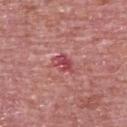lesion size: ≈3 mm; body site: the upper back; subject: male, aged 73 to 77; lighting: white-light illumination; image source: 15 mm crop, total-body photography.